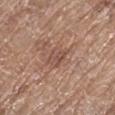The lesion was tiled from a total-body skin photograph and was not biopsied.
Approximately 3.5 mm at its widest.
Captured under white-light illumination.
A female subject, roughly 75 years of age.
Cropped from a whole-body photographic skin survey; the tile spans about 15 mm.
The lesion is on the left lower leg.
An algorithmic analysis of the crop reported an area of roughly 4.5 mm², a shape eccentricity near 0.85, and two-axis asymmetry of about 0.5. The analysis additionally found a lesion color around L≈50 a*≈20 b*≈25 in CIELAB, a lesion–skin lightness drop of about 8, and a normalized border contrast of about 5.5.